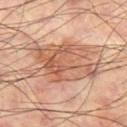The lesion was photographed on a routine skin check and not biopsied; there is no pathology result. This image is a 15 mm lesion crop taken from a total-body photograph. The recorded lesion diameter is about 8 mm. The lesion is on the left thigh. A male patient, aged approximately 60. The lesion-visualizer software estimated an average lesion color of about L≈58 a*≈22 b*≈31 (CIELAB), a lesion–skin lightness drop of about 11, and a lesion-to-skin contrast of about 7 (normalized; higher = more distinct). It also reported a border-irregularity index near 4.5/10, a color-variation rating of about 6/10, and a peripheral color-asymmetry measure near 2.